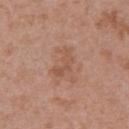Q: Is there a histopathology result?
A: catalogued during a skin exam; not biopsied
Q: How was this image acquired?
A: ~15 mm crop, total-body skin-cancer survey
Q: Patient demographics?
A: female, roughly 40 years of age
Q: What is the anatomic site?
A: the upper back
Q: Lesion size?
A: ~3.5 mm (longest diameter)
Q: Illumination type?
A: white-light illumination